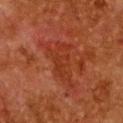Clinical impression:
No biopsy was performed on this lesion — it was imaged during a full skin examination and was not determined to be concerning.